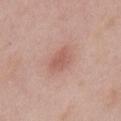<tbp_lesion>
<biopsy_status>not biopsied; imaged during a skin examination</biopsy_status>
<image>
  <source>total-body photography crop</source>
  <field_of_view_mm>15</field_of_view_mm>
</image>
<lighting>white-light</lighting>
<lesion_size>
  <long_diameter_mm_approx>3.5</long_diameter_mm_approx>
</lesion_size>
<site>front of the torso</site>
<patient>
  <sex>male</sex>
  <age_approx>55</age_approx>
</patient>
<automated_metrics>
  <area_mm2_approx>6.0</area_mm2_approx>
  <shape_asymmetry>0.2</shape_asymmetry>
  <cielab_L>58</cielab_L>
  <cielab_a>23</cielab_a>
  <cielab_b>26</cielab_b>
  <vs_skin_darker_L>8.0</vs_skin_darker_L>
  <border_irregularity_0_10>2.0</border_irregularity_0_10>
  <peripheral_color_asymmetry>0.5</peripheral_color_asymmetry>
</automated_metrics>
</tbp_lesion>